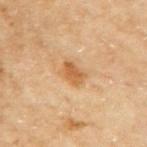workup: total-body-photography surveillance lesion; no biopsy
TBP lesion metrics: a border-irregularity index near 2/10 and a within-lesion color-variation index near 2.5/10; an automated nevus-likeness rating near 85 out of 100 and lesion-presence confidence of about 100/100
acquisition: ~15 mm tile from a whole-body skin photo
tile lighting: cross-polarized
patient: female, in their 60s
body site: the right upper arm
diameter: about 3 mm